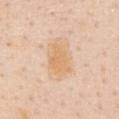Q: Was a biopsy performed?
A: no biopsy performed (imaged during a skin exam)
Q: How large is the lesion?
A: ~5 mm (longest diameter)
Q: What are the patient's age and sex?
A: female, in their 60s
Q: What kind of image is this?
A: ~15 mm crop, total-body skin-cancer survey
Q: Illumination type?
A: white-light
Q: What is the anatomic site?
A: the chest